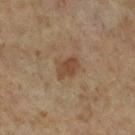| key | value |
|---|---|
| biopsy status | total-body-photography surveillance lesion; no biopsy |
| patient | female, about 65 years old |
| location | the left leg |
| image | total-body-photography crop, ~15 mm field of view |
| lesion diameter | about 3 mm |